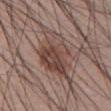<record>
<biopsy_status>not biopsied; imaged during a skin examination</biopsy_status>
<lighting>white-light</lighting>
<automated_metrics>
  <area_mm2_approx>20.0</area_mm2_approx>
  <eccentricity>0.85</eccentricity>
  <shape_asymmetry>0.3</shape_asymmetry>
  <border_irregularity_0_10>5.0</border_irregularity_0_10>
  <peripheral_color_asymmetry>3.5</peripheral_color_asymmetry>
</automated_metrics>
<image>
  <source>total-body photography crop</source>
  <field_of_view_mm>15</field_of_view_mm>
</image>
<site>abdomen</site>
<patient>
  <sex>male</sex>
  <age_approx>40</age_approx>
</patient>
</record>